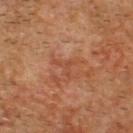{
  "biopsy_status": "not biopsied; imaged during a skin examination",
  "site": "head or neck",
  "image": {
    "source": "total-body photography crop",
    "field_of_view_mm": 15
  },
  "patient": {
    "sex": "male",
    "age_approx": 70
  }
}